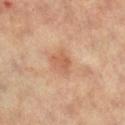No biopsy was performed on this lesion — it was imaged during a full skin examination and was not determined to be concerning. The patient is a female roughly 65 years of age. A region of skin cropped from a whole-body photographic capture, roughly 15 mm wide. The lesion is on the left leg.